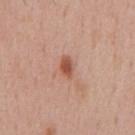The lesion was tiled from a total-body skin photograph and was not biopsied. The lesion is on the chest. A 15 mm crop from a total-body photograph taken for skin-cancer surveillance. Imaged with white-light lighting. The subject is a male in their mid- to late 50s. Approximately 3 mm at its widest.